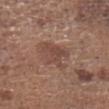A close-up tile cropped from a whole-body skin photograph, about 15 mm across.
The lesion is located on the head or neck.
About 4.5 mm across.
Automated tile analysis of the lesion measured an outline eccentricity of about 0.75 (0 = round, 1 = elongated) and two-axis asymmetry of about 0.45. The software also gave a border-irregularity index near 5.5/10, a within-lesion color-variation index near 2/10, and radial color variation of about 0.5.
This is a white-light tile.
A male subject approximately 75 years of age.